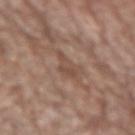Imaged during a routine full-body skin examination; the lesion was not biopsied and no histopathology is available. A male subject in their 70s. The lesion is on the left forearm. The total-body-photography lesion software estimated a mean CIELAB color near L≈48 a*≈18 b*≈26, a lesion–skin lightness drop of about 7, and a normalized border contrast of about 5.5. It also reported a nevus-likeness score of about 0/100 and lesion-presence confidence of about 75/100. Imaged with white-light lighting. A 15 mm close-up extracted from a 3D total-body photography capture. Approximately 3.5 mm at its widest.